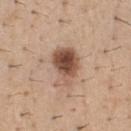Notes:
* notes — no biopsy performed (imaged during a skin exam)
* patient — male, aged 38–42
* automated metrics — border irregularity of about 3 on a 0–10 scale, a within-lesion color-variation index near 8/10, and peripheral color asymmetry of about 3; an automated nevus-likeness rating near 95 out of 100 and a detector confidence of about 100 out of 100 that the crop contains a lesion
* lesion size — about 5 mm
* image source — 15 mm crop, total-body photography
* anatomic site — the chest
* lighting — white-light illumination